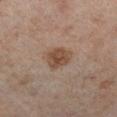Clinical impression: Captured during whole-body skin photography for melanoma surveillance; the lesion was not biopsied. Acquisition and patient details: Approximately 3.5 mm at its widest. A female patient, about 50 years old. On the right lower leg. A lesion tile, about 15 mm wide, cut from a 3D total-body photograph.